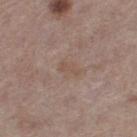workup: total-body-photography surveillance lesion; no biopsy | subject: female, in their 60s | diameter: about 2.5 mm | image-analysis metrics: an area of roughly 2.5 mm², an outline eccentricity of about 0.9 (0 = round, 1 = elongated), and a shape-asymmetry score of about 0.4 (0 = symmetric); an automated nevus-likeness rating near 0 out of 100 and lesion-presence confidence of about 100/100 | imaging modality: total-body-photography crop, ~15 mm field of view | location: the left thigh | tile lighting: white-light illumination.